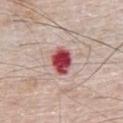biopsy status=no biopsy performed (imaged during a skin exam); site=the front of the torso; lighting=white-light illumination; diameter=~3.5 mm (longest diameter); image=total-body-photography crop, ~15 mm field of view; subject=male, roughly 80 years of age.